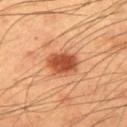The lesion was tiled from a total-body skin photograph and was not biopsied. Longest diameter approximately 3.5 mm. The subject is a male aged around 55. The lesion-visualizer software estimated a footprint of about 9.5 mm² and a shape eccentricity near 0.5. And it measured a border-irregularity index near 1.5/10. The software also gave a nevus-likeness score of about 100/100 and a detector confidence of about 100 out of 100 that the crop contains a lesion. A 15 mm close-up tile from a total-body photography series done for melanoma screening. The tile uses cross-polarized illumination. The lesion is on the right upper arm.